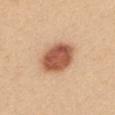<case>
<biopsy_status>not biopsied; imaged during a skin examination</biopsy_status>
<lighting>white-light</lighting>
<image>
  <source>total-body photography crop</source>
  <field_of_view_mm>15</field_of_view_mm>
</image>
<lesion_size>
  <long_diameter_mm_approx>5.5</long_diameter_mm_approx>
</lesion_size>
<patient>
  <sex>female</sex>
  <age_approx>25</age_approx>
</patient>
<site>mid back</site>
</case>